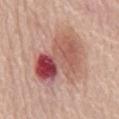Impression: Imaged during a routine full-body skin examination; the lesion was not biopsied and no histopathology is available.